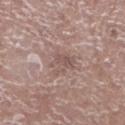The lesion was photographed on a routine skin check and not biopsied; there is no pathology result.
The lesion is located on the leg.
A male patient, about 70 years old.
Automated image analysis of the tile measured a mean CIELAB color near L≈53 a*≈17 b*≈21, roughly 7 lightness units darker than nearby skin, and a lesion-to-skin contrast of about 5.5 (normalized; higher = more distinct). And it measured a border-irregularity rating of about 6/10 and peripheral color asymmetry of about 0.5.
A region of skin cropped from a whole-body photographic capture, roughly 15 mm wide.
The tile uses white-light illumination.